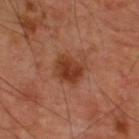Captured during whole-body skin photography for melanoma surveillance; the lesion was not biopsied.
A male patient, approximately 60 years of age.
An algorithmic analysis of the crop reported a lesion area of about 9 mm², a shape eccentricity near 0.5, and two-axis asymmetry of about 0.2. The analysis additionally found a lesion–skin lightness drop of about 10.
About 3.5 mm across.
A 15 mm close-up extracted from a 3D total-body photography capture.
Captured under cross-polarized illumination.
On the upper back.